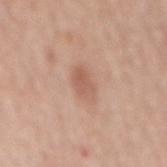biopsy status — total-body-photography surveillance lesion; no biopsy
image source — ~15 mm tile from a whole-body skin photo
patient — male, aged 68 to 72
lesion size — ~3.5 mm (longest diameter)
tile lighting — white-light illumination
body site — the mid back